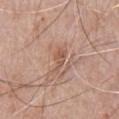{"patient": {"sex": "male", "age_approx": 80}, "site": "chest", "image": {"source": "total-body photography crop", "field_of_view_mm": 15}}